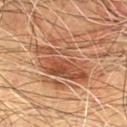Assessment:
The lesion was tiled from a total-body skin photograph and was not biopsied.
Context:
On the chest. The lesion's longest dimension is about 7 mm. A 15 mm close-up tile from a total-body photography series done for melanoma screening. The total-body-photography lesion software estimated an area of roughly 19 mm² and a shape eccentricity near 0.8. It also reported a border-irregularity rating of about 7.5/10, a color-variation rating of about 5.5/10, and a peripheral color-asymmetry measure near 2. The analysis additionally found a detector confidence of about 100 out of 100 that the crop contains a lesion. The subject is a male aged 58 to 62.